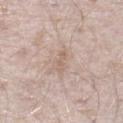– workup: catalogued during a skin exam; not biopsied
– imaging modality: total-body-photography crop, ~15 mm field of view
– anatomic site: the left lower leg
– lesion diameter: ~3 mm (longest diameter)
– patient: male, roughly 40 years of age
– lighting: white-light